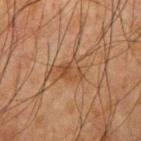biopsy status = catalogued during a skin exam; not biopsied | subject = male, aged 63–67 | tile lighting = cross-polarized | image = total-body-photography crop, ~15 mm field of view | site = the right upper arm.